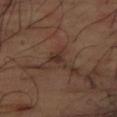| key | value |
|---|---|
| workup | imaged on a skin check; not biopsied |
| image | ~15 mm tile from a whole-body skin photo |
| automated metrics | a mean CIELAB color near L≈31 a*≈16 b*≈22, roughly 7 lightness units darker than nearby skin, and a normalized border contrast of about 7; a border-irregularity rating of about 5.5/10; a classifier nevus-likeness of about 0/100 |
| patient | male, aged 63–67 |
| diameter | ≈3 mm |
| tile lighting | cross-polarized illumination |
| site | the right thigh |